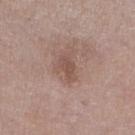Clinical impression: The lesion was tiled from a total-body skin photograph and was not biopsied. Clinical summary: Imaged with white-light lighting. Measured at roughly 3 mm in maximum diameter. Located on the right lower leg. A roughly 15 mm field-of-view crop from a total-body skin photograph. A male patient aged 68–72.